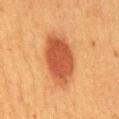Case summary:
* biopsy status · no biopsy performed (imaged during a skin exam)
* automated metrics · a footprint of about 20 mm² and two-axis asymmetry of about 0.2; a mean CIELAB color near L≈45 a*≈26 b*≈35 and roughly 13 lightness units darker than nearby skin; a nevus-likeness score of about 100/100 and a lesion-detection confidence of about 100/100
* diameter · ≈7 mm
* illumination · cross-polarized
* image source · ~15 mm tile from a whole-body skin photo
* anatomic site · the mid back
* subject · female, about 50 years old